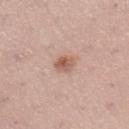image source — ~15 mm crop, total-body skin-cancer survey | subject — female, aged around 25 | lighting — white-light | site — the left lower leg | lesion diameter — ~2.5 mm (longest diameter).